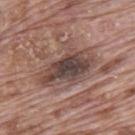Case summary:
– biopsy status · no biopsy performed (imaged during a skin exam)
– body site · the mid back
– TBP lesion metrics · a lesion area of about 20 mm² and a shape eccentricity near 0.8; a mean CIELAB color near L≈44 a*≈16 b*≈21, a lesion–skin lightness drop of about 12, and a normalized border contrast of about 9.5
– lighting · white-light illumination
– imaging modality · ~15 mm crop, total-body skin-cancer survey
– subject · male, about 70 years old